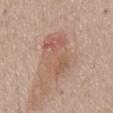Q: Is there a histopathology result?
A: imaged on a skin check; not biopsied
Q: What is the anatomic site?
A: the chest
Q: What are the patient's age and sex?
A: male, aged 73 to 77
Q: What is the lesion's diameter?
A: about 7 mm
Q: What lighting was used for the tile?
A: white-light
Q: What is the imaging modality?
A: 15 mm crop, total-body photography
Q: Automated lesion metrics?
A: a lesion color around L≈58 a*≈19 b*≈28 in CIELAB and a lesion–skin lightness drop of about 8; a border-irregularity index near 2.5/10 and a peripheral color-asymmetry measure near 2